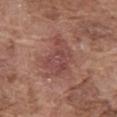Q: Was this lesion biopsied?
A: no biopsy performed (imaged during a skin exam)
Q: Patient demographics?
A: male, aged approximately 75
Q: What did automated image analysis measure?
A: an area of roughly 13 mm², an eccentricity of roughly 0.7, and two-axis asymmetry of about 0.35; an automated nevus-likeness rating near 0 out of 100
Q: What kind of image is this?
A: ~15 mm crop, total-body skin-cancer survey
Q: Lesion location?
A: the abdomen
Q: Illumination type?
A: white-light